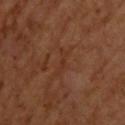  biopsy_status: not biopsied; imaged during a skin examination
  site: upper back
  lighting: cross-polarized
  image:
    source: total-body photography crop
    field_of_view_mm: 15
  patient:
    sex: male
    age_approx: 65
  automated_metrics:
    cielab_L: 31
    cielab_a: 22
    cielab_b: 29
    vs_skin_contrast_norm: 5.0
    border_irregularity_0_10: 7.0
    peripheral_color_asymmetry: 0.0
    nevus_likeness_0_100: 0
    lesion_detection_confidence_0_100: 95